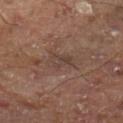image-analysis metrics = a within-lesion color-variation index near 4/10 and peripheral color asymmetry of about 1.5; an automated nevus-likeness rating near 0 out of 100 and lesion-presence confidence of about 90/100 | body site = the left thigh | acquisition = total-body-photography crop, ~15 mm field of view | lesion size = ~3 mm (longest diameter).